The patient is a male approximately 55 years of age · a close-up tile cropped from a whole-body skin photograph, about 15 mm across · the lesion is on the leg — 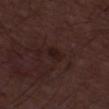The tile uses white-light illumination. Approximately 2.5 mm at its widest. The biopsy diagnosis was a benign skin lesion: dermatofibroma.